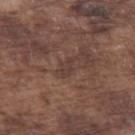Q: Was a biopsy performed?
A: no biopsy performed (imaged during a skin exam)
Q: Lesion location?
A: the left forearm
Q: Lesion size?
A: about 2.5 mm
Q: How was this image acquired?
A: 15 mm crop, total-body photography
Q: What lighting was used for the tile?
A: white-light illumination
Q: Who is the patient?
A: male, about 75 years old